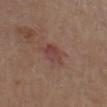Image and clinical context:
Captured under white-light illumination. The lesion is located on the chest. The recorded lesion diameter is about 3.5 mm. This image is a 15 mm lesion crop taken from a total-body photograph. A male subject, aged 68 to 72. Automated tile analysis of the lesion measured an automated nevus-likeness rating near 0 out of 100 and a lesion-detection confidence of about 100/100.